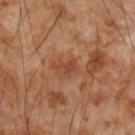follow-up: catalogued during a skin exam; not biopsied | image: 15 mm crop, total-body photography | automated lesion analysis: an average lesion color of about L≈45 a*≈25 b*≈34 (CIELAB), a lesion–skin lightness drop of about 7, and a normalized border contrast of about 6; a border-irregularity index near 5/10, a within-lesion color-variation index near 1.5/10, and radial color variation of about 0.5; a classifier nevus-likeness of about 0/100 and a detector confidence of about 100 out of 100 that the crop contains a lesion | subject: male, aged 53–57 | lesion size: about 2.5 mm | site: the right forearm.